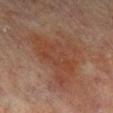notes: catalogued during a skin exam; not biopsied
patient: female, approximately 80 years of age
lighting: cross-polarized
image source: ~15 mm crop, total-body skin-cancer survey
location: the right lower leg